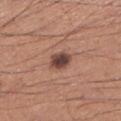Imaged during a routine full-body skin examination; the lesion was not biopsied and no histopathology is available. From the left lower leg. Cropped from a total-body skin-imaging series; the visible field is about 15 mm. The patient is a male aged approximately 55.